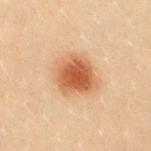{
  "biopsy_status": "not biopsied; imaged during a skin examination",
  "lesion_size": {
    "long_diameter_mm_approx": 4.5
  },
  "lighting": "cross-polarized",
  "image": {
    "source": "total-body photography crop",
    "field_of_view_mm": 15
  },
  "site": "chest",
  "automated_metrics": {
    "area_mm2_approx": 15.0,
    "shape_asymmetry": 0.15,
    "cielab_L": 46,
    "cielab_a": 20,
    "cielab_b": 31,
    "vs_skin_contrast_norm": 9.0,
    "border_irregularity_0_10": 2.0,
    "color_variation_0_10": 4.5,
    "peripheral_color_asymmetry": 1.0
  },
  "patient": {
    "sex": "female",
    "age_approx": 20
  }
}